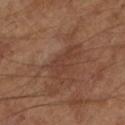Assessment: Recorded during total-body skin imaging; not selected for excision or biopsy. Background: A close-up tile cropped from a whole-body skin photograph, about 15 mm across. The patient is a male aged around 65. This is a cross-polarized tile. The recorded lesion diameter is about 4 mm.